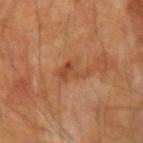Q: Was this lesion biopsied?
A: catalogued during a skin exam; not biopsied
Q: Patient demographics?
A: male, aged approximately 65
Q: Lesion size?
A: about 4 mm
Q: What lighting was used for the tile?
A: cross-polarized illumination
Q: Lesion location?
A: the right upper arm
Q: How was this image acquired?
A: ~15 mm tile from a whole-body skin photo